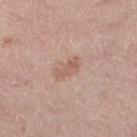workup: total-body-photography surveillance lesion; no biopsy | image source: total-body-photography crop, ~15 mm field of view | anatomic site: the right thigh | subject: female, roughly 55 years of age.